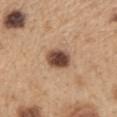  image:
    source: total-body photography crop
    field_of_view_mm: 15
  patient:
    sex: female
    age_approx: 60
  site: upper back